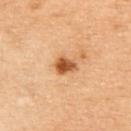Captured during whole-body skin photography for melanoma surveillance; the lesion was not biopsied. Located on the upper back. Cropped from a total-body skin-imaging series; the visible field is about 15 mm. The patient is a female aged 58–62.